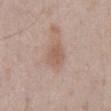No biopsy was performed on this lesion — it was imaged during a full skin examination and was not determined to be concerning. Located on the abdomen. Captured under white-light illumination. A male subject, in their 50s. A region of skin cropped from a whole-body photographic capture, roughly 15 mm wide. About 3 mm across.